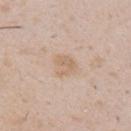Impression: The lesion was tiled from a total-body skin photograph and was not biopsied. Context: A lesion tile, about 15 mm wide, cut from a 3D total-body photograph. A male subject roughly 50 years of age. About 3 mm across. This is a white-light tile. The lesion is located on the right upper arm.